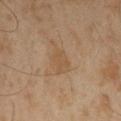follow-up: catalogued during a skin exam; not biopsied | image-analysis metrics: an outline eccentricity of about 0.7 (0 = round, 1 = elongated) and two-axis asymmetry of about 0.35; internal color variation of about 0.5 on a 0–10 scale and a peripheral color-asymmetry measure near 0; a nevus-likeness score of about 0/100 and a lesion-detection confidence of about 100/100 | image source: ~15 mm tile from a whole-body skin photo | subject: male, aged 58 to 62 | location: the left thigh | illumination: cross-polarized.